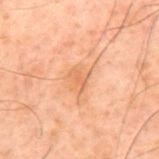Clinical impression:
No biopsy was performed on this lesion — it was imaged during a full skin examination and was not determined to be concerning.
Acquisition and patient details:
The lesion's longest dimension is about 2.5 mm. A 15 mm close-up tile from a total-body photography series done for melanoma screening. A male patient aged approximately 60. From the mid back. The tile uses cross-polarized illumination.